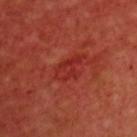follow-up = catalogued during a skin exam; not biopsied.